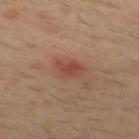biopsy status: no biopsy performed (imaged during a skin exam)
TBP lesion metrics: a lesion area of about 5 mm², a shape eccentricity near 0.6, and a symmetry-axis asymmetry near 0.25; a lesion color around L≈44 a*≈26 b*≈25 in CIELAB, about 8 CIELAB-L* units darker than the surrounding skin, and a lesion-to-skin contrast of about 6.5 (normalized; higher = more distinct); a border-irregularity rating of about 3/10; a nevus-likeness score of about 0/100 and lesion-presence confidence of about 100/100
anatomic site: the mid back
diameter: ~2.5 mm (longest diameter)
subject: male, in their 30s
illumination: cross-polarized illumination
acquisition: 15 mm crop, total-body photography